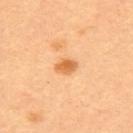follow-up — total-body-photography surveillance lesion; no biopsy
site — the upper back
lesion diameter — ≈2.5 mm
imaging modality — ~15 mm crop, total-body skin-cancer survey
illumination — cross-polarized
automated metrics — a lesion color around L≈64 a*≈26 b*≈46 in CIELAB and a lesion-to-skin contrast of about 8 (normalized; higher = more distinct); a border-irregularity rating of about 1.5/10, a color-variation rating of about 2.5/10, and radial color variation of about 1; a classifier nevus-likeness of about 95/100
subject — female, aged approximately 35